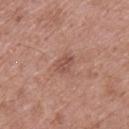notes: total-body-photography surveillance lesion; no biopsy | image: ~15 mm tile from a whole-body skin photo | patient: male, aged around 65 | image-analysis metrics: an outline eccentricity of about 0.75 (0 = round, 1 = elongated); a nevus-likeness score of about 0/100 | anatomic site: the upper back | size: ~2.5 mm (longest diameter).